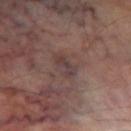Notes:
* biopsy status — imaged on a skin check; not biopsied
* illumination — cross-polarized illumination
* patient — male, approximately 70 years of age
* site — the left thigh
* size — about 3 mm
* image source — ~15 mm tile from a whole-body skin photo